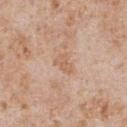biopsy status = no biopsy performed (imaged during a skin exam); patient = male, aged around 65; anatomic site = the chest; imaging modality = ~15 mm crop, total-body skin-cancer survey; illumination = white-light.